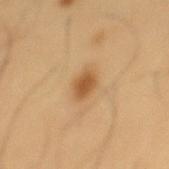Captured during whole-body skin photography for melanoma surveillance; the lesion was not biopsied. Captured under cross-polarized illumination. The lesion's longest dimension is about 3 mm. Located on the mid back. The subject is a male aged around 55. Cropped from a total-body skin-imaging series; the visible field is about 15 mm. The lesion-visualizer software estimated a lesion color around L≈53 a*≈20 b*≈39 in CIELAB, a lesion–skin lightness drop of about 11, and a normalized border contrast of about 8.5. And it measured border irregularity of about 1.5 on a 0–10 scale, a color-variation rating of about 2/10, and radial color variation of about 0.5.